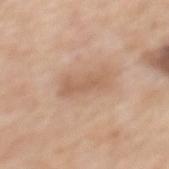Assessment:
Imaged during a routine full-body skin examination; the lesion was not biopsied and no histopathology is available.
Image and clinical context:
A roughly 15 mm field-of-view crop from a total-body skin photograph. Located on the mid back. A female patient aged 63–67. Captured under white-light illumination. The recorded lesion diameter is about 4.5 mm.